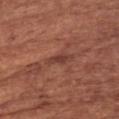Case summary:
• biopsy status — no biopsy performed (imaged during a skin exam)
• imaging modality — ~15 mm crop, total-body skin-cancer survey
• lighting — white-light
• body site — the chest
• subject — male, in their mid-60s
• diameter — ≈3 mm